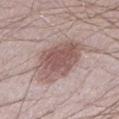Part of a total-body skin-imaging series; this lesion was reviewed on a skin check and was not flagged for biopsy. On the left lower leg. A 15 mm close-up extracted from a 3D total-body photography capture. A male patient approximately 25 years of age.